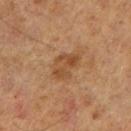Assessment:
The lesion was photographed on a routine skin check and not biopsied; there is no pathology result.
Image and clinical context:
This image is a 15 mm lesion crop taken from a total-body photograph. A male patient, roughly 65 years of age. From the right forearm. The tile uses cross-polarized illumination. Automated image analysis of the tile measured a shape eccentricity near 0.8 and a shape-asymmetry score of about 0.3 (0 = symmetric). The analysis additionally found a normalized lesion–skin contrast near 6.5. The software also gave an automated nevus-likeness rating near 0 out of 100.